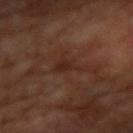<record>
  <biopsy_status>not biopsied; imaged during a skin examination</biopsy_status>
  <patient>
    <sex>male</sex>
    <age_approx>70</age_approx>
  </patient>
  <site>right upper arm</site>
  <image>
    <source>total-body photography crop</source>
    <field_of_view_mm>15</field_of_view_mm>
  </image>
  <automated_metrics>
    <border_irregularity_0_10>7.0</border_irregularity_0_10>
    <color_variation_0_10>2.0</color_variation_0_10>
    <peripheral_color_asymmetry>0.5</peripheral_color_asymmetry>
  </automated_metrics>
  <lighting>cross-polarized</lighting>
</record>